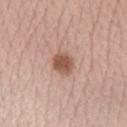Q: Is there a histopathology result?
A: total-body-photography surveillance lesion; no biopsy
Q: Automated lesion metrics?
A: a mean CIELAB color near L≈54 a*≈22 b*≈28 and about 13 CIELAB-L* units darker than the surrounding skin; internal color variation of about 2.5 on a 0–10 scale and a peripheral color-asymmetry measure near 1
Q: How large is the lesion?
A: about 2.5 mm
Q: What is the imaging modality?
A: ~15 mm tile from a whole-body skin photo
Q: Lesion location?
A: the right forearm
Q: Patient demographics?
A: female, about 40 years old
Q: Illumination type?
A: white-light illumination